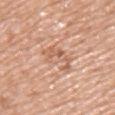The lesion was tiled from a total-body skin photograph and was not biopsied. A male subject, in their mid-50s. This image is a 15 mm lesion crop taken from a total-body photograph. From the arm. The tile uses white-light illumination.